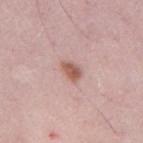Recorded during total-body skin imaging; not selected for excision or biopsy. Captured under white-light illumination. The lesion is located on the right upper arm. The total-body-photography lesion software estimated border irregularity of about 2.5 on a 0–10 scale, a within-lesion color-variation index near 3/10, and radial color variation of about 1. It also reported an automated nevus-likeness rating near 95 out of 100 and a lesion-detection confidence of about 100/100. Longest diameter approximately 2.5 mm. Cropped from a whole-body photographic skin survey; the tile spans about 15 mm. A male subject, about 50 years old.